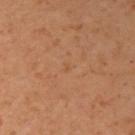The lesion was tiled from a total-body skin photograph and was not biopsied.
Imaged with cross-polarized lighting.
A female subject, aged approximately 40.
A roughly 15 mm field-of-view crop from a total-body skin photograph.
On the arm.
The lesion's longest dimension is about 1.5 mm.